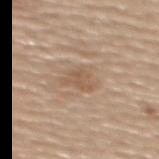Findings:
– workup — catalogued during a skin exam; not biopsied
– subject — female, in their 60s
– illumination — white-light
– acquisition — ~15 mm tile from a whole-body skin photo
– body site — the upper back
– size — ≈3.5 mm